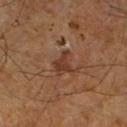Clinical impression:
The lesion was tiled from a total-body skin photograph and was not biopsied.
Clinical summary:
A male subject, about 65 years old. The lesion is on the left lower leg. The recorded lesion diameter is about 3 mm. The lesion-visualizer software estimated a border-irregularity rating of about 5/10, a color-variation rating of about 2/10, and a peripheral color-asymmetry measure near 0.5. Imaged with cross-polarized lighting. A 15 mm close-up extracted from a 3D total-body photography capture.